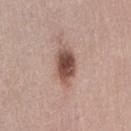workup: imaged on a skin check; not biopsied | size: ~4.5 mm (longest diameter) | image source: ~15 mm tile from a whole-body skin photo | subject: female, aged around 40 | TBP lesion metrics: an area of roughly 9.5 mm² and an outline eccentricity of about 0.8 (0 = round, 1 = elongated); a lesion color around L≈50 a*≈19 b*≈25 in CIELAB and roughly 15 lightness units darker than nearby skin | illumination: white-light | site: the lower back.